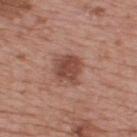Imaged during a routine full-body skin examination; the lesion was not biopsied and no histopathology is available. This image is a 15 mm lesion crop taken from a total-body photograph. From the upper back. The subject is a male aged 73–77. The recorded lesion diameter is about 3.5 mm. Automated image analysis of the tile measured a lesion area of about 8 mm² and a symmetry-axis asymmetry near 0.25. It also reported roughly 12 lightness units darker than nearby skin and a normalized border contrast of about 8.5. The analysis additionally found a within-lesion color-variation index near 3.5/10 and radial color variation of about 1.